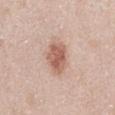| key | value |
|---|---|
| follow-up | no biopsy performed (imaged during a skin exam) |
| patient | female, aged approximately 50 |
| anatomic site | the right thigh |
| acquisition | ~15 mm crop, total-body skin-cancer survey |